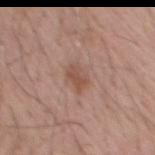Clinical impression:
Part of a total-body skin-imaging series; this lesion was reviewed on a skin check and was not flagged for biopsy.